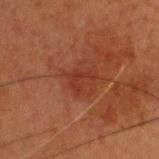The lesion was tiled from a total-body skin photograph and was not biopsied. The recorded lesion diameter is about 2.5 mm. On the head or neck. The lesion-visualizer software estimated a lesion area of about 4 mm², an eccentricity of roughly 0.7, and two-axis asymmetry of about 0.6. It also reported a classifier nevus-likeness of about 5/100 and a detector confidence of about 100 out of 100 that the crop contains a lesion. A close-up tile cropped from a whole-body skin photograph, about 15 mm across. A male patient, in their mid-70s.